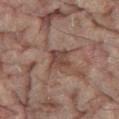This lesion was catalogued during total-body skin photography and was not selected for biopsy. The lesion-visualizer software estimated a lesion area of about 5.5 mm², a shape eccentricity near 0.55, and a symmetry-axis asymmetry near 0.25. It also reported a mean CIELAB color near L≈38 a*≈17 b*≈21 and a lesion–skin lightness drop of about 8. It also reported border irregularity of about 3 on a 0–10 scale and radial color variation of about 1. And it measured an automated nevus-likeness rating near 0 out of 100. The patient is a female aged approximately 80. On the leg. Approximately 3 mm at its widest. Cropped from a whole-body photographic skin survey; the tile spans about 15 mm. The tile uses cross-polarized illumination.